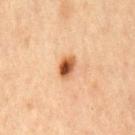<record>
  <biopsy_status>not biopsied; imaged during a skin examination</biopsy_status>
  <patient>
    <sex>male</sex>
    <age_approx>65</age_approx>
  </patient>
  <site>chest</site>
  <image>
    <source>total-body photography crop</source>
    <field_of_view_mm>15</field_of_view_mm>
  </image>
</record>